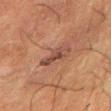{"biopsy_status": "not biopsied; imaged during a skin examination", "lighting": "cross-polarized", "site": "right forearm", "automated_metrics": {"eccentricity": 0.9, "border_irregularity_0_10": 5.0, "color_variation_0_10": 2.5, "peripheral_color_asymmetry": 1.0, "nevus_likeness_0_100": 0}, "patient": {"sex": "male", "age_approx": 60}, "image": {"source": "total-body photography crop", "field_of_view_mm": 15}, "lesion_size": {"long_diameter_mm_approx": 4.0}}